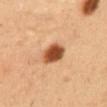Part of a total-body skin-imaging series; this lesion was reviewed on a skin check and was not flagged for biopsy.
The recorded lesion diameter is about 3 mm.
The lesion is located on the abdomen.
A close-up tile cropped from a whole-body skin photograph, about 15 mm across.
Captured under cross-polarized illumination.
A male patient about 50 years old.
Automated tile analysis of the lesion measured a lesion color around L≈50 a*≈27 b*≈39 in CIELAB and about 20 CIELAB-L* units darker than the surrounding skin. The software also gave border irregularity of about 1.5 on a 0–10 scale and internal color variation of about 4.5 on a 0–10 scale. It also reported a nevus-likeness score of about 100/100 and lesion-presence confidence of about 100/100.